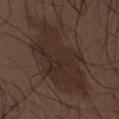Q: Lesion location?
A: the upper back
Q: What are the patient's age and sex?
A: male, aged around 30
Q: Lesion size?
A: ~11 mm (longest diameter)
Q: How was this image acquired?
A: 15 mm crop, total-body photography